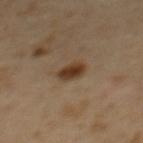Q: Was a biopsy performed?
A: catalogued during a skin exam; not biopsied
Q: Lesion location?
A: the mid back
Q: How large is the lesion?
A: ~3 mm (longest diameter)
Q: What kind of image is this?
A: 15 mm crop, total-body photography
Q: What are the patient's age and sex?
A: female, about 55 years old